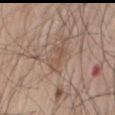Findings:
* follow-up · catalogued during a skin exam; not biopsied
* subject · male, approximately 70 years of age
* location · the mid back
* lighting · white-light
* image · ~15 mm tile from a whole-body skin photo